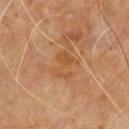Assessment: No biopsy was performed on this lesion — it was imaged during a full skin examination and was not determined to be concerning. Clinical summary: The lesion's longest dimension is about 3.5 mm. The patient is a male approximately 60 years of age. A 15 mm close-up extracted from a 3D total-body photography capture. The total-body-photography lesion software estimated an area of roughly 6 mm², an outline eccentricity of about 0.8 (0 = round, 1 = elongated), and a shape-asymmetry score of about 0.35 (0 = symmetric). The software also gave an automated nevus-likeness rating near 0 out of 100 and a detector confidence of about 100 out of 100 that the crop contains a lesion. Located on the upper back.